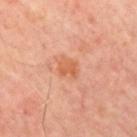Longest diameter approximately 2.5 mm. Cropped from a total-body skin-imaging series; the visible field is about 15 mm. Automated tile analysis of the lesion measured an area of roughly 4.5 mm², an eccentricity of roughly 0.65, and a shape-asymmetry score of about 0.35 (0 = symmetric). The analysis additionally found a lesion color around L≈60 a*≈28 b*≈38 in CIELAB, a lesion–skin lightness drop of about 8, and a normalized border contrast of about 6.5. The analysis additionally found a nevus-likeness score of about 5/100 and a detector confidence of about 100 out of 100 that the crop contains a lesion. A patient roughly 55 years of age. On the mid back.